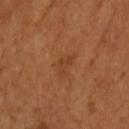Q: Was this lesion biopsied?
A: no biopsy performed (imaged during a skin exam)
Q: What are the patient's age and sex?
A: female, roughly 55 years of age
Q: What is the anatomic site?
A: the right forearm
Q: How large is the lesion?
A: about 3 mm
Q: What kind of image is this?
A: total-body-photography crop, ~15 mm field of view
Q: How was the tile lit?
A: cross-polarized illumination